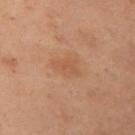Assessment:
This lesion was catalogued during total-body skin photography and was not selected for biopsy.
Acquisition and patient details:
A female subject in their mid- to late 50s. The lesion is on the chest. Automated tile analysis of the lesion measured an area of roughly 5 mm², a shape eccentricity near 0.65, and two-axis asymmetry of about 0.35. The analysis additionally found border irregularity of about 3.5 on a 0–10 scale and peripheral color asymmetry of about 0.5. This image is a 15 mm lesion crop taken from a total-body photograph. Longest diameter approximately 3 mm. The tile uses cross-polarized illumination.